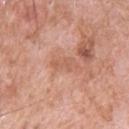The lesion was tiled from a total-body skin photograph and was not biopsied.